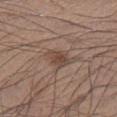Part of a total-body skin-imaging series; this lesion was reviewed on a skin check and was not flagged for biopsy.
Imaged with white-light lighting.
On the leg.
A close-up tile cropped from a whole-body skin photograph, about 15 mm across.
A male patient aged approximately 35.
Measured at roughly 2.5 mm in maximum diameter.